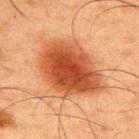notes: catalogued during a skin exam; not biopsied | subject: male, aged 58–62 | acquisition: total-body-photography crop, ~15 mm field of view | body site: the upper back | lesion diameter: ~8 mm (longest diameter) | tile lighting: cross-polarized | TBP lesion metrics: two-axis asymmetry of about 0.2; a within-lesion color-variation index near 5.5/10 and peripheral color asymmetry of about 1.5; a detector confidence of about 100 out of 100 that the crop contains a lesion.